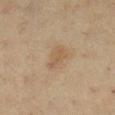Imaged during a routine full-body skin examination; the lesion was not biopsied and no histopathology is available. Located on the right lower leg. The total-body-photography lesion software estimated an area of roughly 4.5 mm². And it measured a border-irregularity rating of about 4/10, a color-variation rating of about 2/10, and a peripheral color-asymmetry measure near 0.5. And it measured a classifier nevus-likeness of about 20/100 and lesion-presence confidence of about 100/100. The subject is a female approximately 50 years of age. A 15 mm close-up extracted from a 3D total-body photography capture. Imaged with cross-polarized lighting.